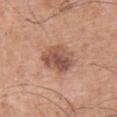* follow-up: catalogued during a skin exam; not biopsied
* subject: male, roughly 65 years of age
* image source: total-body-photography crop, ~15 mm field of view
* location: the chest